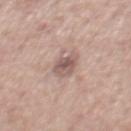Assessment:
Imaged during a routine full-body skin examination; the lesion was not biopsied and no histopathology is available.
Clinical summary:
Imaged with white-light lighting. A roughly 15 mm field-of-view crop from a total-body skin photograph. The lesion's longest dimension is about 3 mm. The subject is a male aged 48 to 52. The total-body-photography lesion software estimated a lesion area of about 5.5 mm², an outline eccentricity of about 0.5 (0 = round, 1 = elongated), and a symmetry-axis asymmetry near 0.25. The analysis additionally found a mean CIELAB color near L≈56 a*≈17 b*≈21, about 11 CIELAB-L* units darker than the surrounding skin, and a lesion-to-skin contrast of about 7.5 (normalized; higher = more distinct). It also reported a within-lesion color-variation index near 4.5/10 and peripheral color asymmetry of about 1.5. The software also gave an automated nevus-likeness rating near 5 out of 100 and lesion-presence confidence of about 100/100. The lesion is on the chest.